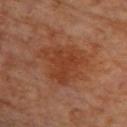This lesion was catalogued during total-body skin photography and was not selected for biopsy. A 15 mm close-up extracted from a 3D total-body photography capture. A female patient, aged around 65. The recorded lesion diameter is about 6.5 mm. On the upper back. Captured under cross-polarized illumination.